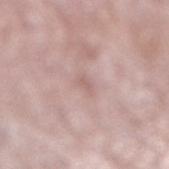Assessment: Captured during whole-body skin photography for melanoma surveillance; the lesion was not biopsied. Acquisition and patient details: A lesion tile, about 15 mm wide, cut from a 3D total-body photograph. A female patient in their mid-70s. This is a white-light tile. The lesion is on the right lower leg.